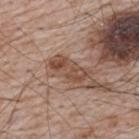Assessment: The lesion was photographed on a routine skin check and not biopsied; there is no pathology result.